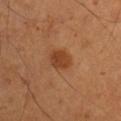notes: no biopsy performed (imaged during a skin exam); TBP lesion metrics: an area of roughly 6.5 mm²; diameter: about 3 mm; image: 15 mm crop, total-body photography; anatomic site: the right upper arm; tile lighting: cross-polarized illumination; subject: male, aged 53 to 57.